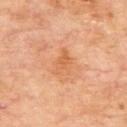Notes:
• workup — imaged on a skin check; not biopsied
• tile lighting — cross-polarized
• subject — male, in their 70s
• site — the upper back
• acquisition — ~15 mm crop, total-body skin-cancer survey
• automated lesion analysis — an area of roughly 5.5 mm² and a shape-asymmetry score of about 0.35 (0 = symmetric); a color-variation rating of about 3/10 and peripheral color asymmetry of about 1; an automated nevus-likeness rating near 0 out of 100 and a lesion-detection confidence of about 100/100
• lesion size — ≈3.5 mm